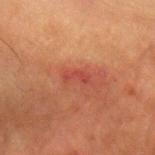<tbp_lesion>
<biopsy_status>not biopsied; imaged during a skin examination</biopsy_status>
<patient>
  <sex>male</sex>
  <age_approx>65</age_approx>
</patient>
<image>
  <source>total-body photography crop</source>
  <field_of_view_mm>15</field_of_view_mm>
</image>
<lesion_size>
  <long_diameter_mm_approx>3.0</long_diameter_mm_approx>
</lesion_size>
<site>arm</site>
</tbp_lesion>